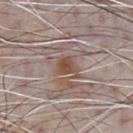Clinical impression:
Part of a total-body skin-imaging series; this lesion was reviewed on a skin check and was not flagged for biopsy.
Clinical summary:
The tile uses white-light illumination. The lesion's longest dimension is about 2.5 mm. The lesion is on the chest. A 15 mm close-up tile from a total-body photography series done for melanoma screening. The patient is a male in their 70s. An algorithmic analysis of the crop reported a footprint of about 4.5 mm², a shape eccentricity near 0.45, and a shape-asymmetry score of about 0.3 (0 = symmetric).